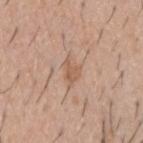Findings:
– notes: no biopsy performed (imaged during a skin exam)
– location: the upper back
– image: total-body-photography crop, ~15 mm field of view
– tile lighting: white-light illumination
– TBP lesion metrics: a lesion area of about 4 mm², an outline eccentricity of about 0.8 (0 = round, 1 = elongated), and a shape-asymmetry score of about 0.45 (0 = symmetric); a lesion color around L≈58 a*≈19 b*≈31 in CIELAB, about 8 CIELAB-L* units darker than the surrounding skin, and a normalized lesion–skin contrast near 6; a classifier nevus-likeness of about 0/100 and a lesion-detection confidence of about 100/100
– subject: male, roughly 60 years of age